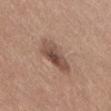No biopsy was performed on this lesion — it was imaged during a full skin examination and was not determined to be concerning. The subject is a female approximately 35 years of age. On the right thigh. A roughly 15 mm field-of-view crop from a total-body skin photograph.